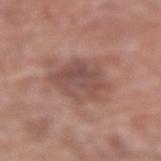notes: total-body-photography surveillance lesion; no biopsy
subject: female, approximately 80 years of age
acquisition: ~15 mm crop, total-body skin-cancer survey
tile lighting: white-light
site: the left forearm